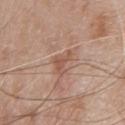Captured during whole-body skin photography for melanoma surveillance; the lesion was not biopsied. A male subject, aged 78–82. A region of skin cropped from a whole-body photographic capture, roughly 15 mm wide. Automated image analysis of the tile measured about 8 CIELAB-L* units darker than the surrounding skin and a normalized border contrast of about 6. The lesion is on the chest. Longest diameter approximately 3 mm.